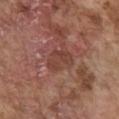  biopsy_status: not biopsied; imaged during a skin examination
  patient:
    sex: male
    age_approx: 75
  image:
    source: total-body photography crop
    field_of_view_mm: 15
  site: chest
  lesion_size:
    long_diameter_mm_approx: 3.5
  lighting: white-light